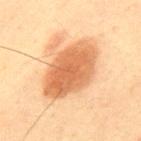Recorded during total-body skin imaging; not selected for excision or biopsy. On the mid back. Imaged with cross-polarized lighting. Cropped from a whole-body photographic skin survey; the tile spans about 15 mm. A male subject aged 58–62.